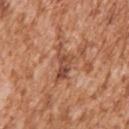biopsy_status: not biopsied; imaged during a skin examination
lighting: white-light
patient:
  sex: male
  age_approx: 45
lesion_size:
  long_diameter_mm_approx: 4.5
site: right upper arm
image:
  source: total-body photography crop
  field_of_view_mm: 15